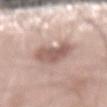Case summary:
– workup — imaged on a skin check; not biopsied
– lesion diameter — ≈5.5 mm
– subject — male, about 70 years old
– lighting — white-light illumination
– body site — the mid back
– TBP lesion metrics — a lesion color around L≈58 a*≈18 b*≈23 in CIELAB and a lesion–skin lightness drop of about 12; a border-irregularity rating of about 4/10, internal color variation of about 3.5 on a 0–10 scale, and peripheral color asymmetry of about 1
– image source — 15 mm crop, total-body photography